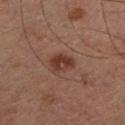Imaged during a routine full-body skin examination; the lesion was not biopsied and no histopathology is available.
The lesion is on the left lower leg.
A male patient about 60 years old.
An algorithmic analysis of the crop reported a classifier nevus-likeness of about 80/100 and a lesion-detection confidence of about 100/100.
A close-up tile cropped from a whole-body skin photograph, about 15 mm across.